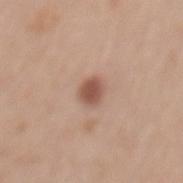  biopsy_status: not biopsied; imaged during a skin examination
  lighting: white-light
  patient:
    sex: male
    age_approx: 65
  lesion_size:
    long_diameter_mm_approx: 2.5
  automated_metrics:
    nevus_likeness_0_100: 95
    lesion_detection_confidence_0_100: 100
  site: mid back
  image:
    source: total-body photography crop
    field_of_view_mm: 15